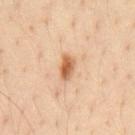Recorded during total-body skin imaging; not selected for excision or biopsy.
On the chest.
A male patient aged approximately 35.
About 3 mm across.
A 15 mm close-up tile from a total-body photography series done for melanoma screening.
Automated image analysis of the tile measured a footprint of about 4.5 mm², an outline eccentricity of about 0.85 (0 = round, 1 = elongated), and a symmetry-axis asymmetry near 0.25. It also reported a mean CIELAB color near L≈56 a*≈21 b*≈35, roughly 13 lightness units darker than nearby skin, and a lesion-to-skin contrast of about 9 (normalized; higher = more distinct).